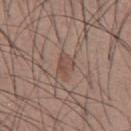This lesion was catalogued during total-body skin photography and was not selected for biopsy. Captured under white-light illumination. Located on the chest. Automated image analysis of the tile measured a footprint of about 5 mm² and a symmetry-axis asymmetry near 0.3. It also reported a nevus-likeness score of about 0/100 and a lesion-detection confidence of about 90/100. A 15 mm crop from a total-body photograph taken for skin-cancer surveillance. A male subject about 35 years old.